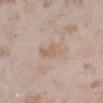Q: Was this lesion biopsied?
A: catalogued during a skin exam; not biopsied
Q: Where on the body is the lesion?
A: the left lower leg
Q: What is the imaging modality?
A: ~15 mm tile from a whole-body skin photo
Q: Automated lesion metrics?
A: a lesion area of about 5.5 mm², an eccentricity of roughly 0.9, and a symmetry-axis asymmetry near 0.35; a lesion color around L≈62 a*≈16 b*≈28 in CIELAB and roughly 7 lightness units darker than nearby skin; a border-irregularity rating of about 3.5/10 and radial color variation of about 1
Q: What are the patient's age and sex?
A: female, aged approximately 25